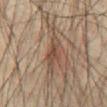The lesion was photographed on a routine skin check and not biopsied; there is no pathology result. Cropped from a total-body skin-imaging series; the visible field is about 15 mm. The lesion is on the abdomen. A male subject about 75 years old. Captured under cross-polarized illumination.